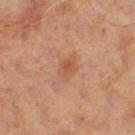This lesion was catalogued during total-body skin photography and was not selected for biopsy. This is a cross-polarized tile. A male patient in their 60s. Approximately 2.5 mm at its widest. The lesion is located on the left thigh. Automated image analysis of the tile measured a shape eccentricity near 0.7. And it measured a mean CIELAB color near L≈50 a*≈24 b*≈32 and a normalized lesion–skin contrast near 5.5. The analysis additionally found a detector confidence of about 100 out of 100 that the crop contains a lesion. A 15 mm crop from a total-body photograph taken for skin-cancer surveillance.